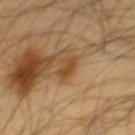notes: no biopsy performed (imaged during a skin exam); anatomic site: the abdomen; imaging modality: total-body-photography crop, ~15 mm field of view; subject: male, aged approximately 60.